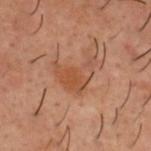This lesion was catalogued during total-body skin photography and was not selected for biopsy. A male patient about 50 years old. The lesion is located on the head or neck. This is a cross-polarized tile. A region of skin cropped from a whole-body photographic capture, roughly 15 mm wide. Automated image analysis of the tile measured a lesion color around L≈41 a*≈20 b*≈28 in CIELAB, a lesion–skin lightness drop of about 6, and a lesion-to-skin contrast of about 6 (normalized; higher = more distinct). It also reported a border-irregularity rating of about 6.5/10, a within-lesion color-variation index near 3.5/10, and peripheral color asymmetry of about 1.